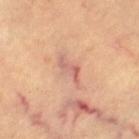No biopsy was performed on this lesion — it was imaged during a full skin examination and was not determined to be concerning. A subject approximately 60 years of age. Cropped from a whole-body photographic skin survey; the tile spans about 15 mm. The lesion is on the leg.